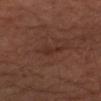Impression: Part of a total-body skin-imaging series; this lesion was reviewed on a skin check and was not flagged for biopsy. Image and clinical context: Approximately 3 mm at its widest. A male subject aged 63–67. On the left upper arm. The tile uses cross-polarized illumination. A 15 mm close-up tile from a total-body photography series done for melanoma screening.